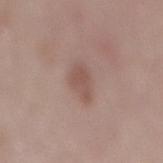Recorded during total-body skin imaging; not selected for excision or biopsy. A male patient, aged 68–72. This image is a 15 mm lesion crop taken from a total-body photograph. Located on the mid back.